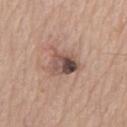{
  "biopsy_status": "not biopsied; imaged during a skin examination",
  "image": {
    "source": "total-body photography crop",
    "field_of_view_mm": 15
  },
  "site": "front of the torso",
  "patient": {
    "sex": "male",
    "age_approx": 80
  }
}